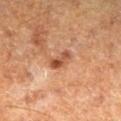<record>
  <biopsy_status>not biopsied; imaged during a skin examination</biopsy_status>
  <site>left lower leg</site>
  <lighting>cross-polarized</lighting>
  <lesion_size>
    <long_diameter_mm_approx>3.0</long_diameter_mm_approx>
  </lesion_size>
  <image>
    <source>total-body photography crop</source>
    <field_of_view_mm>15</field_of_view_mm>
  </image>
  <automated_metrics>
    <area_mm2_approx>4.0</area_mm2_approx>
    <shape_asymmetry>0.3</shape_asymmetry>
    <border_irregularity_0_10>3.0</border_irregularity_0_10>
    <color_variation_0_10>5.5</color_variation_0_10>
    <peripheral_color_asymmetry>1.5</peripheral_color_asymmetry>
    <nevus_likeness_0_100>0</nevus_likeness_0_100>
    <lesion_detection_confidence_0_100>100</lesion_detection_confidence_0_100>
  </automated_metrics>
  <patient>
    <sex>male</sex>
    <age_approx>60</age_approx>
  </patient>
</record>